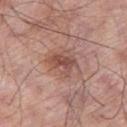Q: Was a biopsy performed?
A: catalogued during a skin exam; not biopsied
Q: What are the patient's age and sex?
A: male, about 70 years old
Q: What is the imaging modality?
A: total-body-photography crop, ~15 mm field of view
Q: Where on the body is the lesion?
A: the right thigh
Q: Lesion size?
A: ~4.5 mm (longest diameter)